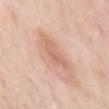Q: Is there a histopathology result?
A: total-body-photography surveillance lesion; no biopsy
Q: What lighting was used for the tile?
A: white-light
Q: Who is the patient?
A: female, aged around 50
Q: Automated lesion metrics?
A: a shape eccentricity near 0.8 and two-axis asymmetry of about 0.4; border irregularity of about 4 on a 0–10 scale, a within-lesion color-variation index near 3/10, and radial color variation of about 1; a lesion-detection confidence of about 100/100
Q: What is the anatomic site?
A: the right upper arm
Q: What kind of image is this?
A: total-body-photography crop, ~15 mm field of view
Q: How large is the lesion?
A: about 3.5 mm